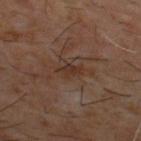Assessment:
No biopsy was performed on this lesion — it was imaged during a full skin examination and was not determined to be concerning.
Acquisition and patient details:
An algorithmic analysis of the crop reported a mean CIELAB color near L≈30 a*≈16 b*≈23, about 6 CIELAB-L* units darker than the surrounding skin, and a lesion-to-skin contrast of about 6.5 (normalized; higher = more distinct). It also reported a border-irregularity index near 3/10, internal color variation of about 1.5 on a 0–10 scale, and peripheral color asymmetry of about 0.5. A lesion tile, about 15 mm wide, cut from a 3D total-body photograph. The recorded lesion diameter is about 3 mm. Captured under cross-polarized illumination. Located on the upper back. A male patient approximately 60 years of age.